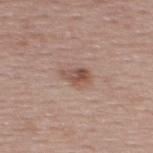Q: Is there a histopathology result?
A: total-body-photography surveillance lesion; no biopsy
Q: Lesion size?
A: ~3 mm (longest diameter)
Q: What is the anatomic site?
A: the upper back
Q: What are the patient's age and sex?
A: female, in their mid- to late 50s
Q: Illumination type?
A: white-light
Q: How was this image acquired?
A: total-body-photography crop, ~15 mm field of view